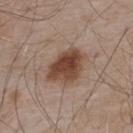{"biopsy_status": "not biopsied; imaged during a skin examination", "automated_metrics": {"cielab_L": 44, "cielab_a": 19, "cielab_b": 27, "vs_skin_darker_L": 14.0, "vs_skin_contrast_norm": 10.5, "color_variation_0_10": 5.0, "peripheral_color_asymmetry": 1.5}, "image": {"source": "total-body photography crop", "field_of_view_mm": 15}, "site": "upper back", "lighting": "white-light", "patient": {"sex": "male", "age_approx": 55}}